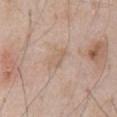  lesion_size:
    long_diameter_mm_approx: 3.0
  lighting: white-light
  patient:
    sex: male
    age_approx: 60
  automated_metrics:
    vs_skin_darker_L: 6.0
  image:
    source: total-body photography crop
    field_of_view_mm: 15
  site: abdomen